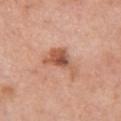| feature | finding |
|---|---|
| notes | total-body-photography surveillance lesion; no biopsy |
| automated metrics | a lesion area of about 8 mm² and an eccentricity of roughly 0.85; roughly 12 lightness units darker than nearby skin; an automated nevus-likeness rating near 65 out of 100 and lesion-presence confidence of about 100/100 |
| image | ~15 mm crop, total-body skin-cancer survey |
| subject | female, in their mid-70s |
| anatomic site | the chest |